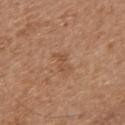<case>
<biopsy_status>not biopsied; imaged during a skin examination</biopsy_status>
<site>upper back</site>
<image>
  <source>total-body photography crop</source>
  <field_of_view_mm>15</field_of_view_mm>
</image>
<patient>
  <sex>male</sex>
  <age_approx>70</age_approx>
</patient>
</case>